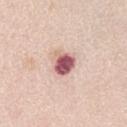| key | value |
|---|---|
| workup | no biopsy performed (imaged during a skin exam) |
| image source | 15 mm crop, total-body photography |
| lighting | white-light |
| location | the abdomen |
| patient | female, aged 63 to 67 |